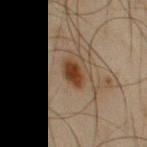Captured during whole-body skin photography for melanoma surveillance; the lesion was not biopsied.
A lesion tile, about 15 mm wide, cut from a 3D total-body photograph.
The tile uses cross-polarized illumination.
A male subject approximately 50 years of age.
Longest diameter approximately 3.5 mm.
On the right upper arm.
Automated tile analysis of the lesion measured a footprint of about 5.5 mm² and a shape-asymmetry score of about 0.2 (0 = symmetric). The software also gave an average lesion color of about L≈30 a*≈15 b*≈25 (CIELAB) and a normalized border contrast of about 10.5. The analysis additionally found border irregularity of about 1.5 on a 0–10 scale, a within-lesion color-variation index near 3.5/10, and a peripheral color-asymmetry measure near 1. The software also gave a classifier nevus-likeness of about 95/100 and lesion-presence confidence of about 100/100.